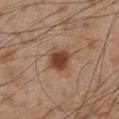notes: catalogued during a skin exam; not biopsied | subject: male, in their mid-50s | acquisition: ~15 mm crop, total-body skin-cancer survey | tile lighting: cross-polarized illumination | location: the left lower leg.